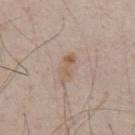The lesion was tiled from a total-body skin photograph and was not biopsied. A roughly 15 mm field-of-view crop from a total-body skin photograph. This is a white-light tile. The patient is a male roughly 50 years of age. The lesion is on the front of the torso.